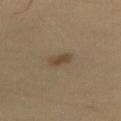Q: What lighting was used for the tile?
A: cross-polarized
Q: How was this image acquired?
A: ~15 mm tile from a whole-body skin photo
Q: What are the patient's age and sex?
A: male, roughly 65 years of age
Q: What did automated image analysis measure?
A: a footprint of about 4 mm², an outline eccentricity of about 0.85 (0 = round, 1 = elongated), and a shape-asymmetry score of about 0.2 (0 = symmetric)
Q: Lesion size?
A: ~3 mm (longest diameter)